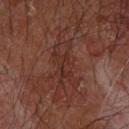- follow-up: no biopsy performed (imaged during a skin exam)
- image-analysis metrics: an eccentricity of roughly 0.9; a lesion color around L≈29 a*≈21 b*≈22 in CIELAB, a lesion–skin lightness drop of about 5, and a lesion-to-skin contrast of about 5 (normalized; higher = more distinct); a nevus-likeness score of about 0/100 and lesion-presence confidence of about 60/100
- lesion size: about 5.5 mm
- anatomic site: the arm
- illumination: cross-polarized illumination
- acquisition: 15 mm crop, total-body photography
- patient: male, aged 63 to 67